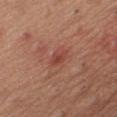From the right thigh. A 15 mm crop from a total-body photograph taken for skin-cancer surveillance. A female subject, aged around 50.